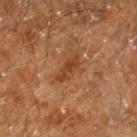Q: How was the tile lit?
A: cross-polarized
Q: How large is the lesion?
A: ~3.5 mm (longest diameter)
Q: How was this image acquired?
A: ~15 mm tile from a whole-body skin photo
Q: What is the anatomic site?
A: the right lower leg
Q: Who is the patient?
A: male, approximately 60 years of age